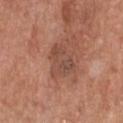follow-up = catalogued during a skin exam; not biopsied